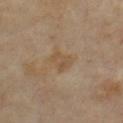Assessment: The lesion was tiled from a total-body skin photograph and was not biopsied. Acquisition and patient details: The subject is a male in their 70s. A 15 mm close-up extracted from a 3D total-body photography capture. Longest diameter approximately 2.5 mm. The tile uses cross-polarized illumination. The lesion is on the chest.